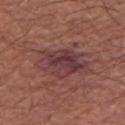Q: Was a biopsy performed?
A: catalogued during a skin exam; not biopsied
Q: Where on the body is the lesion?
A: the left upper arm
Q: What are the patient's age and sex?
A: male, aged around 65
Q: Lesion size?
A: about 6 mm
Q: How was this image acquired?
A: 15 mm crop, total-body photography
Q: What lighting was used for the tile?
A: white-light